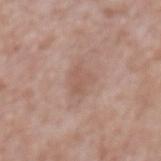Captured during whole-body skin photography for melanoma surveillance; the lesion was not biopsied. Approximately 4 mm at its widest. Cropped from a total-body skin-imaging series; the visible field is about 15 mm. A male patient, about 45 years old. This is a white-light tile. The lesion is located on the mid back.